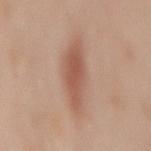A 15 mm close-up tile from a total-body photography series done for melanoma screening.
The patient is a female aged approximately 50.
Located on the mid back.